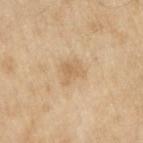Context: This image is a 15 mm lesion crop taken from a total-body photograph. On the right upper arm. A male patient about 70 years old.